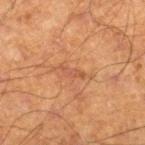Imaged during a routine full-body skin examination; the lesion was not biopsied and no histopathology is available. Captured under cross-polarized illumination. A region of skin cropped from a whole-body photographic capture, roughly 15 mm wide. A male patient, approximately 70 years of age. Automated image analysis of the tile measured a shape eccentricity near 0.9. The analysis additionally found a mean CIELAB color near L≈45 a*≈22 b*≈31, a lesion–skin lightness drop of about 6, and a normalized border contrast of about 5. The analysis additionally found an automated nevus-likeness rating near 0 out of 100 and lesion-presence confidence of about 90/100. From the right lower leg.